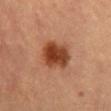The lesion was tiled from a total-body skin photograph and was not biopsied. The lesion is located on the abdomen. A lesion tile, about 15 mm wide, cut from a 3D total-body photograph. A female patient, approximately 60 years of age. Automated image analysis of the tile measured an area of roughly 12 mm². It also reported a nevus-likeness score of about 100/100 and a lesion-detection confidence of about 100/100. Captured under cross-polarized illumination.